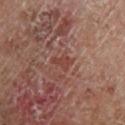The lesion was tiled from a total-body skin photograph and was not biopsied.
The total-body-photography lesion software estimated a mean CIELAB color near L≈40 a*≈24 b*≈25, about 6 CIELAB-L* units darker than the surrounding skin, and a normalized lesion–skin contrast near 6. The software also gave a border-irregularity rating of about 4/10, a within-lesion color-variation index near 1/10, and radial color variation of about 0.
The lesion is located on the left lower leg.
The patient is a male aged 68–72.
A lesion tile, about 15 mm wide, cut from a 3D total-body photograph.
The lesion's longest dimension is about 2.5 mm.
The tile uses cross-polarized illumination.